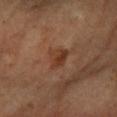Q: Was a biopsy performed?
A: imaged on a skin check; not biopsied
Q: What is the anatomic site?
A: the left forearm
Q: Automated lesion metrics?
A: a mean CIELAB color near L≈36 a*≈21 b*≈29, roughly 7 lightness units darker than nearby skin, and a normalized border contrast of about 6.5; a border-irregularity index near 3/10, a within-lesion color-variation index near 4/10, and peripheral color asymmetry of about 1.5; a classifier nevus-likeness of about 65/100 and a detector confidence of about 100 out of 100 that the crop contains a lesion
Q: What kind of image is this?
A: 15 mm crop, total-body photography
Q: What are the patient's age and sex?
A: female, aged 63 to 67
Q: What is the lesion's diameter?
A: ~3 mm (longest diameter)
Q: What lighting was used for the tile?
A: cross-polarized